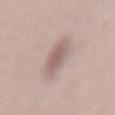Clinical impression: Part of a total-body skin-imaging series; this lesion was reviewed on a skin check and was not flagged for biopsy. Clinical summary: A close-up tile cropped from a whole-body skin photograph, about 15 mm across. An algorithmic analysis of the crop reported a lesion area of about 9.5 mm² and a shape eccentricity near 0.85. Approximately 5 mm at its widest. Located on the mid back. A female subject about 40 years old. This is a white-light tile.